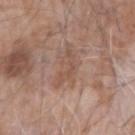Clinical impression: Part of a total-body skin-imaging series; this lesion was reviewed on a skin check and was not flagged for biopsy. Image and clinical context: The lesion is located on the arm. A 15 mm crop from a total-body photograph taken for skin-cancer surveillance. A male patient aged approximately 75.